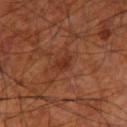The lesion is on the right thigh.
A roughly 15 mm field-of-view crop from a total-body skin photograph.
The patient is a male aged 68–72.